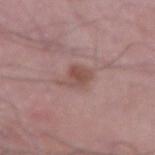Imaged during a routine full-body skin examination; the lesion was not biopsied and no histopathology is available.
A 15 mm close-up tile from a total-body photography series done for melanoma screening.
This is a white-light tile.
On the right upper arm.
Automated image analysis of the tile measured a lesion area of about 5.5 mm², a shape eccentricity near 0.8, and two-axis asymmetry of about 0.35. The software also gave an average lesion color of about L≈50 a*≈20 b*≈22 (CIELAB) and about 8 CIELAB-L* units darker than the surrounding skin. It also reported border irregularity of about 3.5 on a 0–10 scale, a within-lesion color-variation index near 2.5/10, and radial color variation of about 0.5.
The lesion's longest dimension is about 3.5 mm.
A male subject, aged approximately 50.